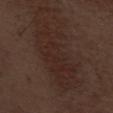Clinical impression:
Imaged during a routine full-body skin examination; the lesion was not biopsied and no histopathology is available.
Background:
A male patient, aged 68–72. A close-up tile cropped from a whole-body skin photograph, about 15 mm across. From the abdomen.